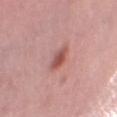Assessment: No biopsy was performed on this lesion — it was imaged during a full skin examination and was not determined to be concerning. Acquisition and patient details: From the chest. A female patient about 40 years old. The tile uses white-light illumination. A 15 mm crop from a total-body photograph taken for skin-cancer surveillance. Longest diameter approximately 2.5 mm.